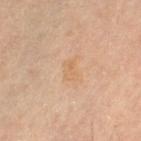Case summary:
- follow-up · catalogued during a skin exam; not biopsied
- location · the left thigh
- patient · female, aged around 60
- imaging modality · ~15 mm crop, total-body skin-cancer survey